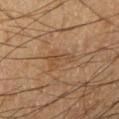Clinical impression:
Imaged during a routine full-body skin examination; the lesion was not biopsied and no histopathology is available.
Context:
Captured under cross-polarized illumination. Automated tile analysis of the lesion measured an area of roughly 5.5 mm² and a shape eccentricity near 0.8. And it measured roughly 5 lightness units darker than nearby skin and a lesion-to-skin contrast of about 5 (normalized; higher = more distinct). And it measured border irregularity of about 6 on a 0–10 scale, internal color variation of about 2.5 on a 0–10 scale, and a peripheral color-asymmetry measure near 1. And it measured lesion-presence confidence of about 95/100. Located on the right forearm. A male subject, aged approximately 60. About 3.5 mm across. A lesion tile, about 15 mm wide, cut from a 3D total-body photograph.